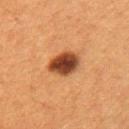Case summary:
• workup — total-body-photography surveillance lesion; no biopsy
• diameter — about 3.5 mm
• site — the right upper arm
• illumination — cross-polarized illumination
• imaging modality — ~15 mm crop, total-body skin-cancer survey
• subject — female, aged approximately 40